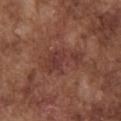Assessment:
Part of a total-body skin-imaging series; this lesion was reviewed on a skin check and was not flagged for biopsy.
Context:
Located on the chest. This is a white-light tile. The lesion's longest dimension is about 6 mm. A male subject in their mid-70s. A 15 mm close-up tile from a total-body photography series done for melanoma screening.